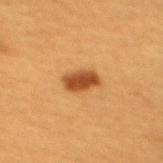workup — catalogued during a skin exam; not biopsied | imaging modality — ~15 mm crop, total-body skin-cancer survey | lighting — cross-polarized | lesion size — ≈3.5 mm | site — the mid back | patient — female, about 30 years old.